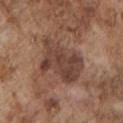Notes:
* notes: no biopsy performed (imaged during a skin exam)
* lesion diameter: ≈6.5 mm
* subject: male, aged 73–77
* tile lighting: white-light
* automated lesion analysis: a footprint of about 20 mm², an outline eccentricity of about 0.8 (0 = round, 1 = elongated), and a shape-asymmetry score of about 0.25 (0 = symmetric); an automated nevus-likeness rating near 15 out of 100 and a lesion-detection confidence of about 100/100
* image source: total-body-photography crop, ~15 mm field of view
* site: the arm